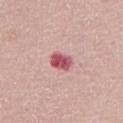{"biopsy_status": "not biopsied; imaged during a skin examination", "image": {"source": "total-body photography crop", "field_of_view_mm": 15}, "patient": {"sex": "female", "age_approx": 65}, "automated_metrics": {"area_mm2_approx": 5.5, "eccentricity": 0.75, "shape_asymmetry": 0.15, "cielab_L": 55, "cielab_a": 33, "cielab_b": 19, "vs_skin_darker_L": 16.0, "border_irregularity_0_10": 1.5, "color_variation_0_10": 4.5}, "lighting": "white-light", "site": "abdomen"}